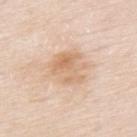Assessment:
This lesion was catalogued during total-body skin photography and was not selected for biopsy.
Image and clinical context:
A male patient, in their mid- to late 70s. Imaged with white-light lighting. A roughly 15 mm field-of-view crop from a total-body skin photograph. The lesion is on the back.